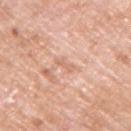Assessment: This lesion was catalogued during total-body skin photography and was not selected for biopsy. Background: The lesion is located on the arm. The subject is a male aged around 60. The lesion's longest dimension is about 2.5 mm. Imaged with white-light lighting. A roughly 15 mm field-of-view crop from a total-body skin photograph.